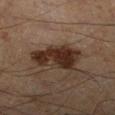Q: Was a biopsy performed?
A: imaged on a skin check; not biopsied
Q: Lesion location?
A: the leg
Q: Lesion size?
A: about 5.5 mm
Q: Illumination type?
A: cross-polarized illumination
Q: How was this image acquired?
A: ~15 mm crop, total-body skin-cancer survey
Q: What did automated image analysis measure?
A: a lesion area of about 13 mm², a shape eccentricity near 0.85, and a symmetry-axis asymmetry near 0.35; a mean CIELAB color near L≈26 a*≈16 b*≈23 and about 13 CIELAB-L* units darker than the surrounding skin; a border-irregularity index near 4.5/10 and a color-variation rating of about 3.5/10
Q: Who is the patient?
A: male, aged 53–57